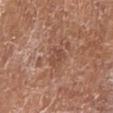| field | value |
|---|---|
| biopsy status | no biopsy performed (imaged during a skin exam) |
| image | total-body-photography crop, ~15 mm field of view |
| lighting | white-light illumination |
| subject | female, aged around 75 |
| site | the left lower leg |